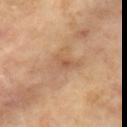| feature | finding |
|---|---|
| workup | no biopsy performed (imaged during a skin exam) |
| image source | total-body-photography crop, ~15 mm field of view |
| illumination | cross-polarized |
| image-analysis metrics | an eccentricity of roughly 0.75 and a shape-asymmetry score of about 0.4 (0 = symmetric); about 7 CIELAB-L* units darker than the surrounding skin and a normalized border contrast of about 4.5 |
| size | ~5 mm (longest diameter) |
| site | the right upper arm |
| patient | female, aged 73–77 |